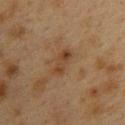Notes:
- biopsy status · total-body-photography surveillance lesion; no biopsy
- imaging modality · 15 mm crop, total-body photography
- site · the back
- lesion diameter · ≈3.5 mm
- tile lighting · cross-polarized
- subject · female, approximately 40 years of age
- automated lesion analysis · two-axis asymmetry of about 0.2; a normalized lesion–skin contrast near 7; a classifier nevus-likeness of about 5/100 and lesion-presence confidence of about 100/100